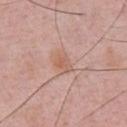Q: Was this lesion biopsied?
A: imaged on a skin check; not biopsied
Q: How was this image acquired?
A: total-body-photography crop, ~15 mm field of view
Q: Who is the patient?
A: male, roughly 50 years of age
Q: Lesion size?
A: ~2.5 mm (longest diameter)
Q: Lesion location?
A: the front of the torso